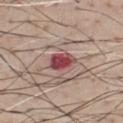Imaged during a routine full-body skin examination; the lesion was not biopsied and no histopathology is available. The lesion's longest dimension is about 3 mm. Located on the chest. This is a white-light tile. A 15 mm crop from a total-body photograph taken for skin-cancer surveillance. A male subject approximately 40 years of age.